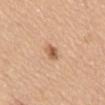Part of a total-body skin-imaging series; this lesion was reviewed on a skin check and was not flagged for biopsy.
A 15 mm close-up tile from a total-body photography series done for melanoma screening.
On the mid back.
A female patient approximately 65 years of age.